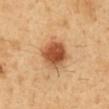Q: Was this lesion biopsied?
A: catalogued during a skin exam; not biopsied
Q: How was the tile lit?
A: cross-polarized
Q: Where on the body is the lesion?
A: the abdomen
Q: Who is the patient?
A: male, about 50 years old
Q: How was this image acquired?
A: ~15 mm tile from a whole-body skin photo